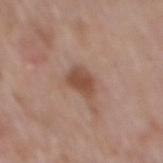<tbp_lesion>
  <biopsy_status>not biopsied; imaged during a skin examination</biopsy_status>
  <image>
    <source>total-body photography crop</source>
    <field_of_view_mm>15</field_of_view_mm>
  </image>
  <site>back</site>
  <lesion_size>
    <long_diameter_mm_approx>3.0</long_diameter_mm_approx>
  </lesion_size>
  <patient>
    <sex>male</sex>
    <age_approx>70</age_approx>
  </patient>
</tbp_lesion>